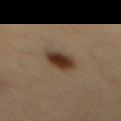This lesion was catalogued during total-body skin photography and was not selected for biopsy. A lesion tile, about 15 mm wide, cut from a 3D total-body photograph. The recorded lesion diameter is about 3.5 mm. Automated tile analysis of the lesion measured an eccentricity of roughly 0.65 and a symmetry-axis asymmetry near 0.2. The software also gave border irregularity of about 2 on a 0–10 scale, internal color variation of about 6 on a 0–10 scale, and radial color variation of about 1.5. This is a cross-polarized tile. The lesion is located on the back. The subject is a male in their mid- to late 30s.